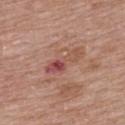diameter: ~4.5 mm (longest diameter) | image: ~15 mm crop, total-body skin-cancer survey | patient: female, approximately 50 years of age | tile lighting: white-light | automated lesion analysis: a footprint of about 6.5 mm², a shape eccentricity near 0.95, and a symmetry-axis asymmetry near 0.45; a lesion color around L≈50 a*≈26 b*≈25 in CIELAB, roughly 9 lightness units darker than nearby skin, and a normalized lesion–skin contrast near 7 | anatomic site: the upper back.